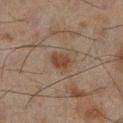Assessment: This lesion was catalogued during total-body skin photography and was not selected for biopsy. Context: The patient is a male about 45 years old. The tile uses cross-polarized illumination. Located on the leg. Approximately 3 mm at its widest. Automated tile analysis of the lesion measured roughly 8 lightness units darker than nearby skin and a normalized border contrast of about 8. The software also gave border irregularity of about 2.5 on a 0–10 scale and radial color variation of about 0.5. A 15 mm crop from a total-body photograph taken for skin-cancer surveillance.